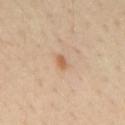Clinical impression: No biopsy was performed on this lesion — it was imaged during a full skin examination and was not determined to be concerning. Context: The patient is a male in their 30s. Automated image analysis of the tile measured a footprint of about 2.5 mm², an eccentricity of roughly 0.75, and a shape-asymmetry score of about 0.2 (0 = symmetric). The analysis additionally found a within-lesion color-variation index near 1/10 and a peripheral color-asymmetry measure near 0.5. The software also gave a nevus-likeness score of about 20/100 and a lesion-detection confidence of about 100/100. The lesion's longest dimension is about 2 mm. From the mid back. A 15 mm crop from a total-body photograph taken for skin-cancer surveillance. The tile uses cross-polarized illumination.